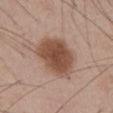Measured at roughly 5.5 mm in maximum diameter. Imaged with white-light lighting. On the abdomen. A male patient approximately 45 years of age. Cropped from a total-body skin-imaging series; the visible field is about 15 mm.